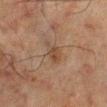Q: Was this lesion biopsied?
A: catalogued during a skin exam; not biopsied
Q: What are the patient's age and sex?
A: male, aged 63–67
Q: Where on the body is the lesion?
A: the right lower leg
Q: What is the imaging modality?
A: 15 mm crop, total-body photography
Q: What did automated image analysis measure?
A: a lesion area of about 4.5 mm², an outline eccentricity of about 0.85 (0 = round, 1 = elongated), and two-axis asymmetry of about 0.35; a lesion color around L≈36 a*≈15 b*≈25 in CIELAB, about 6 CIELAB-L* units darker than the surrounding skin, and a normalized border contrast of about 6; a border-irregularity index near 3.5/10, a color-variation rating of about 2.5/10, and a peripheral color-asymmetry measure near 1
Q: Illumination type?
A: cross-polarized